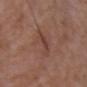<record>
  <biopsy_status>not biopsied; imaged during a skin examination</biopsy_status>
  <image>
    <source>total-body photography crop</source>
    <field_of_view_mm>15</field_of_view_mm>
  </image>
  <patient>
    <sex>male</sex>
    <age_approx>50</age_approx>
  </patient>
  <site>upper back</site>
</record>